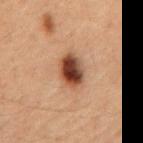The lesion was tiled from a total-body skin photograph and was not biopsied. A 15 mm close-up extracted from a 3D total-body photography capture. On the mid back. A male subject about 65 years old. About 4 mm across. Imaged with cross-polarized lighting.